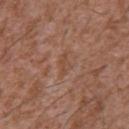Q: What is the lesion's diameter?
A: ~3 mm (longest diameter)
Q: Patient demographics?
A: male, aged 43–47
Q: What lighting was used for the tile?
A: white-light
Q: How was this image acquired?
A: ~15 mm crop, total-body skin-cancer survey
Q: What is the anatomic site?
A: the front of the torso